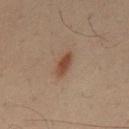Q: Is there a histopathology result?
A: no biopsy performed (imaged during a skin exam)
Q: What is the anatomic site?
A: the mid back
Q: Who is the patient?
A: male, aged 48–52
Q: What kind of image is this?
A: 15 mm crop, total-body photography
Q: Automated lesion metrics?
A: a footprint of about 4 mm², an outline eccentricity of about 0.9 (0 = round, 1 = elongated), and a shape-asymmetry score of about 0.3 (0 = symmetric); a color-variation rating of about 2/10 and radial color variation of about 0.5